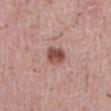An algorithmic analysis of the crop reported an area of roughly 5 mm², a shape eccentricity near 0.55, and a shape-asymmetry score of about 0.15 (0 = symmetric). And it measured a classifier nevus-likeness of about 90/100 and a detector confidence of about 100 out of 100 that the crop contains a lesion.
Imaged with white-light lighting.
Cropped from a whole-body photographic skin survey; the tile spans about 15 mm.
Located on the abdomen.
The subject is a male aged approximately 50.